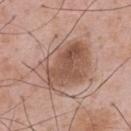Impression:
Part of a total-body skin-imaging series; this lesion was reviewed on a skin check and was not flagged for biopsy.
Background:
A 15 mm crop from a total-body photograph taken for skin-cancer surveillance. Located on the back. This is a white-light tile. Automated image analysis of the tile measured an average lesion color of about L≈54 a*≈19 b*≈27 (CIELAB), roughly 11 lightness units darker than nearby skin, and a normalized border contrast of about 7.5. The patient is a male about 55 years old.